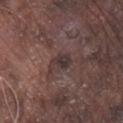A male subject, aged 73–77. Imaged with white-light lighting. The lesion is located on the right lower leg. Cropped from a whole-body photographic skin survey; the tile spans about 15 mm. Approximately 3 mm at its widest.